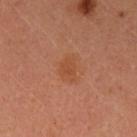The lesion was tiled from a total-body skin photograph and was not biopsied.
Cropped from a whole-body photographic skin survey; the tile spans about 15 mm.
A female subject, aged around 35.
Automated tile analysis of the lesion measured a footprint of about 5 mm², an eccentricity of roughly 0.65, and a shape-asymmetry score of about 0.4 (0 = symmetric). It also reported a mean CIELAB color near L≈47 a*≈25 b*≈35, a lesion–skin lightness drop of about 6, and a normalized border contrast of about 5.5.
Imaged with cross-polarized lighting.
On the left upper arm.